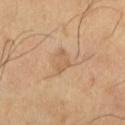<lesion>
  <biopsy_status>not biopsied; imaged during a skin examination</biopsy_status>
  <site>left lower leg</site>
  <automated_metrics>
    <cielab_L>60</cielab_L>
    <cielab_a>17</cielab_a>
    <cielab_b>36</cielab_b>
    <vs_skin_darker_L>7.0</vs_skin_darker_L>
    <border_irregularity_0_10>4.5</border_irregularity_0_10>
    <peripheral_color_asymmetry>0.5</peripheral_color_asymmetry>
  </automated_metrics>
  <patient>
    <sex>male</sex>
    <age_approx>65</age_approx>
  </patient>
  <lighting>cross-polarized</lighting>
  <lesion_size>
    <long_diameter_mm_approx>2.5</long_diameter_mm_approx>
  </lesion_size>
  <image>
    <source>total-body photography crop</source>
    <field_of_view_mm>15</field_of_view_mm>
  </image>
</lesion>